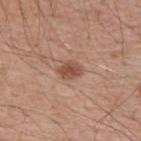Background: A lesion tile, about 15 mm wide, cut from a 3D total-body photograph. Automated tile analysis of the lesion measured a lesion area of about 4.5 mm², an eccentricity of roughly 0.7, and a shape-asymmetry score of about 0.2 (0 = symmetric). It also reported an average lesion color of about L≈50 a*≈21 b*≈29 (CIELAB) and a lesion-to-skin contrast of about 8 (normalized; higher = more distinct). Approximately 3 mm at its widest. The tile uses white-light illumination. The patient is a male aged approximately 55. On the upper back.